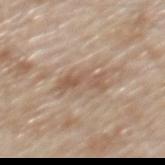No biopsy was performed on this lesion — it was imaged during a full skin examination and was not determined to be concerning.
Cropped from a total-body skin-imaging series; the visible field is about 15 mm.
A male patient roughly 80 years of age.
The lesion is located on the mid back.